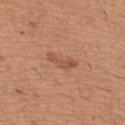This lesion was catalogued during total-body skin photography and was not selected for biopsy.
This image is a 15 mm lesion crop taken from a total-body photograph.
Automated image analysis of the tile measured a lesion-to-skin contrast of about 6 (normalized; higher = more distinct).
Longest diameter approximately 3.5 mm.
The lesion is located on the right upper arm.
The patient is a male roughly 40 years of age.
The tile uses white-light illumination.